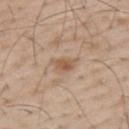Q: What are the patient's age and sex?
A: male, aged around 65
Q: What is the imaging modality?
A: total-body-photography crop, ~15 mm field of view
Q: Lesion location?
A: the upper back